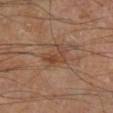Case summary:
– notes · no biopsy performed (imaged during a skin exam)
– patient · male, roughly 70 years of age
– automated lesion analysis · a border-irregularity rating of about 6/10, a within-lesion color-variation index near 3/10, and radial color variation of about 1
– site · the right lower leg
– diameter · ≈3 mm
– illumination · cross-polarized
– image · ~15 mm tile from a whole-body skin photo